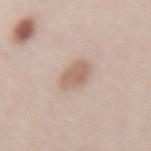Notes:
• biopsy status — imaged on a skin check; not biopsied
• diameter — about 3 mm
• imaging modality — ~15 mm crop, total-body skin-cancer survey
• illumination — white-light
• subject — female, roughly 65 years of age
• location — the lower back